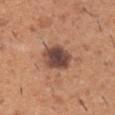Q: Is there a histopathology result?
A: total-body-photography surveillance lesion; no biopsy
Q: Who is the patient?
A: male, aged 38–42
Q: Automated lesion metrics?
A: a footprint of about 10 mm², a shape eccentricity near 0.6, and two-axis asymmetry of about 0.15
Q: What is the anatomic site?
A: the right upper arm
Q: What kind of image is this?
A: 15 mm crop, total-body photography
Q: What lighting was used for the tile?
A: white-light illumination
Q: Lesion size?
A: about 4 mm